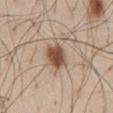{
  "biopsy_status": "not biopsied; imaged during a skin examination",
  "image": {
    "source": "total-body photography crop",
    "field_of_view_mm": 15
  },
  "lesion_size": {
    "long_diameter_mm_approx": 3.5
  },
  "lighting": "white-light",
  "patient": {
    "sex": "male",
    "age_approx": 60
  },
  "site": "abdomen"
}